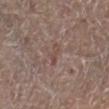This image is a 15 mm lesion crop taken from a total-body photograph.
The lesion is on the leg.
A male subject aged 63 to 67.